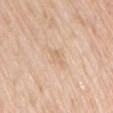No biopsy was performed on this lesion — it was imaged during a full skin examination and was not determined to be concerning.
A female patient about 60 years old.
Automated image analysis of the tile measured an outline eccentricity of about 0.9 (0 = round, 1 = elongated) and a symmetry-axis asymmetry near 0.4.
A lesion tile, about 15 mm wide, cut from a 3D total-body photograph.
The lesion's longest dimension is about 2.5 mm.
From the arm.
This is a white-light tile.